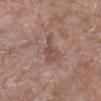Recorded during total-body skin imaging; not selected for excision or biopsy. On the right lower leg. A female subject, aged 73–77. The tile uses white-light illumination. A 15 mm close-up tile from a total-body photography series done for melanoma screening. Longest diameter approximately 3.5 mm.